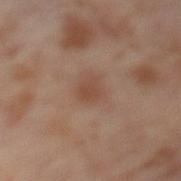<lesion>
  <biopsy_status>not biopsied; imaged during a skin examination</biopsy_status>
  <lighting>cross-polarized</lighting>
  <lesion_size>
    <long_diameter_mm_approx>2.5</long_diameter_mm_approx>
  </lesion_size>
  <site>left thigh</site>
  <image>
    <source>total-body photography crop</source>
    <field_of_view_mm>15</field_of_view_mm>
  </image>
  <automated_metrics>
    <vs_skin_contrast_norm>6.0</vs_skin_contrast_norm>
    <border_irregularity_0_10>2.0</border_irregularity_0_10>
    <color_variation_0_10>1.5</color_variation_0_10>
    <peripheral_color_asymmetry>0.5</peripheral_color_asymmetry>
  </automated_metrics>
  <patient>
    <sex>female</sex>
    <age_approx>55</age_approx>
  </patient>
</lesion>